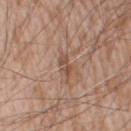Clinical impression:
This lesion was catalogued during total-body skin photography and was not selected for biopsy.
Acquisition and patient details:
Measured at roughly 2.5 mm in maximum diameter. On the arm. This image is a 15 mm lesion crop taken from a total-body photograph. A male subject aged around 60. This is a white-light tile. Automated image analysis of the tile measured an average lesion color of about L≈50 a*≈19 b*≈29 (CIELAB), a lesion–skin lightness drop of about 9, and a normalized border contrast of about 6.5. And it measured lesion-presence confidence of about 85/100.